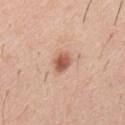This lesion was catalogued during total-body skin photography and was not selected for biopsy. Cropped from a total-body skin-imaging series; the visible field is about 15 mm. The lesion is located on the mid back. The patient is a male in their 40s.